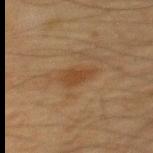follow-up=total-body-photography surveillance lesion; no biopsy | imaging modality=~15 mm tile from a whole-body skin photo | patient=male, aged around 65 | location=the back.